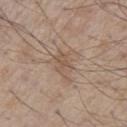notes — no biopsy performed (imaged during a skin exam) | acquisition — ~15 mm crop, total-body skin-cancer survey | image-analysis metrics — a lesion area of about 6.5 mm², a shape eccentricity near 0.75, and two-axis asymmetry of about 0.3; a mean CIELAB color near L≈54 a*≈15 b*≈27, a lesion–skin lightness drop of about 7, and a lesion-to-skin contrast of about 5.5 (normalized; higher = more distinct); a color-variation rating of about 2/10 and a peripheral color-asymmetry measure near 0.5; a classifier nevus-likeness of about 0/100 and lesion-presence confidence of about 100/100 | body site — the chest | subject — male, roughly 80 years of age | illumination — white-light illumination.